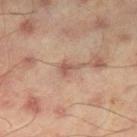follow-up: imaged on a skin check; not biopsied | automated metrics: border irregularity of about 5 on a 0–10 scale and a peripheral color-asymmetry measure near 0.5; a classifier nevus-likeness of about 0/100 and a lesion-detection confidence of about 100/100 | site: the right thigh | lesion diameter: ~3 mm (longest diameter) | image: ~15 mm crop, total-body skin-cancer survey | patient: male, aged approximately 45.